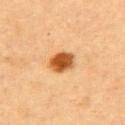Impression: The lesion was tiled from a total-body skin photograph and was not biopsied. Image and clinical context: This is a cross-polarized tile. The subject is a female aged 58–62. Automated image analysis of the tile measured a footprint of about 7 mm² and a symmetry-axis asymmetry near 0.2. It also reported a mean CIELAB color near L≈49 a*≈25 b*≈41, about 17 CIELAB-L* units darker than the surrounding skin, and a normalized lesion–skin contrast near 12. The software also gave a nevus-likeness score of about 100/100 and lesion-presence confidence of about 100/100. A region of skin cropped from a whole-body photographic capture, roughly 15 mm wide. Approximately 3.5 mm at its widest. From the upper back.